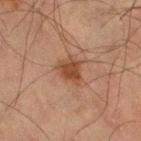Clinical impression:
This lesion was catalogued during total-body skin photography and was not selected for biopsy.
Clinical summary:
Located on the left thigh. A lesion tile, about 15 mm wide, cut from a 3D total-body photograph. About 3 mm across. A male subject approximately 65 years of age. This is a cross-polarized tile.